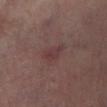Longest diameter approximately 2.5 mm. The tile uses cross-polarized illumination. The total-body-photography lesion software estimated a border-irregularity index near 4/10, a within-lesion color-variation index near 0.5/10, and a peripheral color-asymmetry measure near 0. And it measured a nevus-likeness score of about 0/100 and a detector confidence of about 100 out of 100 that the crop contains a lesion. The patient is a male approximately 65 years of age. The lesion is located on the leg. Cropped from a total-body skin-imaging series; the visible field is about 15 mm.